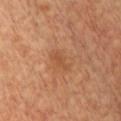The lesion is on the chest.
A male patient about 40 years old.
A roughly 15 mm field-of-view crop from a total-body skin photograph.
The recorded lesion diameter is about 3 mm.
Automated tile analysis of the lesion measured an average lesion color of about L≈51 a*≈24 b*≈37 (CIELAB), about 7 CIELAB-L* units darker than the surrounding skin, and a lesion-to-skin contrast of about 5 (normalized; higher = more distinct). The software also gave a classifier nevus-likeness of about 0/100.